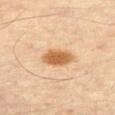  image:
    source: total-body photography crop
    field_of_view_mm: 15
  site: right thigh
  patient:
    sex: male
    age_approx: 60